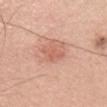Context: Longest diameter approximately 3 mm. The tile uses white-light illumination. Automated tile analysis of the lesion measured an area of roughly 4 mm² and a shape-asymmetry score of about 0.35 (0 = symmetric). The software also gave a nevus-likeness score of about 20/100 and a detector confidence of about 100 out of 100 that the crop contains a lesion. A 15 mm close-up tile from a total-body photography series done for melanoma screening. Located on the head or neck. A female subject, aged approximately 40.